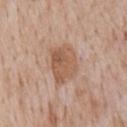biopsy status — imaged on a skin check; not biopsied | anatomic site — the front of the torso | subject — male, about 65 years old | acquisition — total-body-photography crop, ~15 mm field of view.